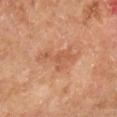Assessment:
Imaged during a routine full-body skin examination; the lesion was not biopsied and no histopathology is available.
Clinical summary:
A male patient aged 63 to 67. Automated tile analysis of the lesion measured an area of roughly 9.5 mm², an eccentricity of roughly 0.9, and two-axis asymmetry of about 0.5. And it measured about 8 CIELAB-L* units darker than the surrounding skin and a normalized border contrast of about 5. The software also gave a border-irregularity rating of about 6.5/10 and internal color variation of about 2.5 on a 0–10 scale. A close-up tile cropped from a whole-body skin photograph, about 15 mm across. Longest diameter approximately 5.5 mm. On the right lower leg.